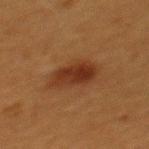| field | value |
|---|---|
| biopsy status | imaged on a skin check; not biopsied |
| image | ~15 mm tile from a whole-body skin photo |
| lighting | cross-polarized illumination |
| patient | female, aged 38 to 42 |
| site | the upper back |
| lesion size | ≈4.5 mm |
| automated metrics | a border-irregularity rating of about 2/10, internal color variation of about 4 on a 0–10 scale, and a peripheral color-asymmetry measure near 1.5 |